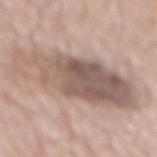follow-up: imaged on a skin check; not biopsied
lesion size: ≈11.5 mm
lighting: white-light illumination
site: the mid back
image source: total-body-photography crop, ~15 mm field of view
patient: male, in their mid- to late 70s